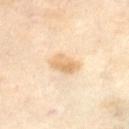Captured during whole-body skin photography for melanoma surveillance; the lesion was not biopsied. This image is a 15 mm lesion crop taken from a total-body photograph. Located on the abdomen. A female subject, aged 53–57. Approximately 3.5 mm at its widest.